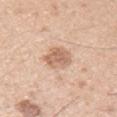No biopsy was performed on this lesion — it was imaged during a full skin examination and was not determined to be concerning. The total-body-photography lesion software estimated border irregularity of about 2 on a 0–10 scale, internal color variation of about 3.5 on a 0–10 scale, and radial color variation of about 1. The analysis additionally found a classifier nevus-likeness of about 60/100 and a lesion-detection confidence of about 100/100. A roughly 15 mm field-of-view crop from a total-body skin photograph. The patient is a male aged around 30. The tile uses white-light illumination. The lesion is located on the arm.